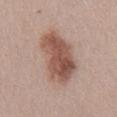workup = no biopsy performed (imaged during a skin exam) | subject = male, in their 40s | imaging modality = 15 mm crop, total-body photography | illumination = white-light illumination | anatomic site = the chest | automated metrics = a symmetry-axis asymmetry near 0.2; a lesion color around L≈53 a*≈20 b*≈25 in CIELAB and a normalized lesion–skin contrast near 9.5; a border-irregularity index near 3/10; an automated nevus-likeness rating near 90 out of 100 and a lesion-detection confidence of about 100/100 | diameter = about 7.5 mm.